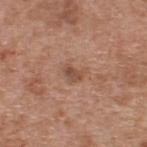Q: Was a biopsy performed?
A: imaged on a skin check; not biopsied
Q: Where on the body is the lesion?
A: the upper back
Q: What lighting was used for the tile?
A: white-light
Q: What is the imaging modality?
A: 15 mm crop, total-body photography
Q: Who is the patient?
A: male, roughly 60 years of age
Q: Lesion size?
A: about 2.5 mm
Q: Automated lesion metrics?
A: an area of roughly 3.5 mm², an outline eccentricity of about 0.7 (0 = round, 1 = elongated), and a symmetry-axis asymmetry near 0.4; an automated nevus-likeness rating near 5 out of 100 and lesion-presence confidence of about 100/100